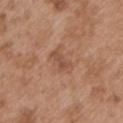Q: Lesion location?
A: the left upper arm
Q: What kind of image is this?
A: total-body-photography crop, ~15 mm field of view
Q: Who is the patient?
A: male, about 55 years old